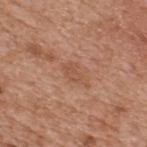Part of a total-body skin-imaging series; this lesion was reviewed on a skin check and was not flagged for biopsy.
A male patient, about 65 years old.
A close-up tile cropped from a whole-body skin photograph, about 15 mm across.
The recorded lesion diameter is about 3 mm.
Automated image analysis of the tile measured an area of roughly 3.5 mm² and two-axis asymmetry of about 0.35. It also reported a lesion color around L≈52 a*≈23 b*≈32 in CIELAB, a lesion–skin lightness drop of about 6, and a lesion-to-skin contrast of about 5 (normalized; higher = more distinct). The analysis additionally found an automated nevus-likeness rating near 0 out of 100 and lesion-presence confidence of about 100/100.
On the upper back.